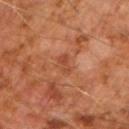The lesion was photographed on a routine skin check and not biopsied; there is no pathology result. A close-up tile cropped from a whole-body skin photograph, about 15 mm across. Located on the right forearm. Longest diameter approximately 2.5 mm. A male subject, about 60 years old.